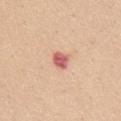workup — imaged on a skin check; not biopsied | lesion size — about 2.5 mm | TBP lesion metrics — an eccentricity of roughly 0.75 and a shape-asymmetry score of about 0.2 (0 = symmetric); an average lesion color of about L≈61 a*≈30 b*≈27 (CIELAB), about 15 CIELAB-L* units darker than the surrounding skin, and a normalized lesion–skin contrast near 9.5; border irregularity of about 2 on a 0–10 scale, internal color variation of about 2.5 on a 0–10 scale, and a peripheral color-asymmetry measure near 1; an automated nevus-likeness rating near 0 out of 100 and lesion-presence confidence of about 100/100 | lighting — white-light illumination | anatomic site — the back | image source — 15 mm crop, total-body photography | subject — female, roughly 45 years of age.